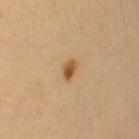Q: Is there a histopathology result?
A: no biopsy performed (imaged during a skin exam)
Q: Patient demographics?
A: female, aged around 30
Q: Where on the body is the lesion?
A: the left upper arm
Q: Illumination type?
A: cross-polarized
Q: What kind of image is this?
A: 15 mm crop, total-body photography
Q: Lesion size?
A: about 2.5 mm